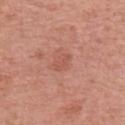Captured during whole-body skin photography for melanoma surveillance; the lesion was not biopsied. This image is a 15 mm lesion crop taken from a total-body photograph. Located on the left upper arm. Automated tile analysis of the lesion measured two-axis asymmetry of about 0.3. The analysis additionally found a mean CIELAB color near L≈55 a*≈27 b*≈29, roughly 6 lightness units darker than nearby skin, and a lesion-to-skin contrast of about 4.5 (normalized; higher = more distinct). It also reported an automated nevus-likeness rating near 0 out of 100 and lesion-presence confidence of about 100/100. The recorded lesion diameter is about 3 mm. A female patient aged around 65.